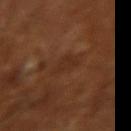Recorded during total-body skin imaging; not selected for excision or biopsy.